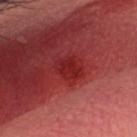biopsy status = catalogued during a skin exam; not biopsied
patient = male, about 35 years old
imaging modality = ~15 mm crop, total-body skin-cancer survey
body site = the head or neck